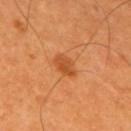Notes:
• workup: total-body-photography surveillance lesion; no biopsy
• lighting: cross-polarized
• image source: total-body-photography crop, ~15 mm field of view
• patient: male, roughly 60 years of age
• location: the mid back